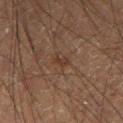Clinical impression: The lesion was tiled from a total-body skin photograph and was not biopsied. Background: The lesion is on the left thigh. Cropped from a total-body skin-imaging series; the visible field is about 15 mm. A male patient, in their 60s. Captured under cross-polarized illumination.